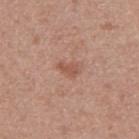Notes:
– biopsy status — total-body-photography surveillance lesion; no biopsy
– size — ≈2.5 mm
– subject — male, aged 48 to 52
– anatomic site — the right upper arm
– TBP lesion metrics — a symmetry-axis asymmetry near 0.35; an automated nevus-likeness rating near 5 out of 100 and a lesion-detection confidence of about 100/100
– image — ~15 mm crop, total-body skin-cancer survey